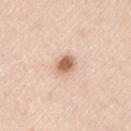<lesion>
  <biopsy_status>not biopsied; imaged during a skin examination</biopsy_status>
  <automated_metrics>
    <area_mm2_approx>5.0</area_mm2_approx>
    <cielab_L>65</cielab_L>
    <cielab_a>20</cielab_a>
    <cielab_b>33</cielab_b>
    <vs_skin_darker_L>15.0</vs_skin_darker_L>
    <vs_skin_contrast_norm>9.0</vs_skin_contrast_norm>
    <border_irregularity_0_10>1.5</border_irregularity_0_10>
    <color_variation_0_10>5.0</color_variation_0_10>
    <peripheral_color_asymmetry>1.5</peripheral_color_asymmetry>
    <nevus_likeness_0_100>100</nevus_likeness_0_100>
    <lesion_detection_confidence_0_100>100</lesion_detection_confidence_0_100>
  </automated_metrics>
  <lighting>white-light</lighting>
  <image>
    <source>total-body photography crop</source>
    <field_of_view_mm>15</field_of_view_mm>
  </image>
  <lesion_size>
    <long_diameter_mm_approx>2.5</long_diameter_mm_approx>
  </lesion_size>
  <site>left upper arm</site>
  <patient>
    <sex>male</sex>
    <age_approx>45</age_approx>
  </patient>
</lesion>